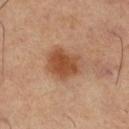follow-up: catalogued during a skin exam; not biopsied | illumination: cross-polarized illumination | TBP lesion metrics: a lesion area of about 13 mm², a shape eccentricity near 0.55, and a shape-asymmetry score of about 0.2 (0 = symmetric); a border-irregularity rating of about 2/10 and a peripheral color-asymmetry measure near 1; a detector confidence of about 100 out of 100 that the crop contains a lesion | site: the right thigh | imaging modality: total-body-photography crop, ~15 mm field of view | subject: female, in their 50s.